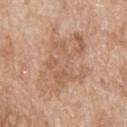Q: Automated lesion metrics?
A: an area of roughly 29 mm², an outline eccentricity of about 0.75 (0 = round, 1 = elongated), and a symmetry-axis asymmetry near 0.35; a mean CIELAB color near L≈60 a*≈19 b*≈31; a border-irregularity rating of about 6.5/10, a within-lesion color-variation index near 5.5/10, and peripheral color asymmetry of about 2; lesion-presence confidence of about 100/100
Q: What are the patient's age and sex?
A: male, about 80 years old
Q: Lesion location?
A: the back
Q: What is the imaging modality?
A: ~15 mm tile from a whole-body skin photo
Q: Lesion size?
A: ≈8 mm